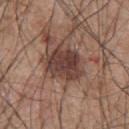Q: Was a biopsy performed?
A: total-body-photography surveillance lesion; no biopsy
Q: What is the lesion's diameter?
A: ≈4.5 mm
Q: What lighting was used for the tile?
A: white-light
Q: Where on the body is the lesion?
A: the upper back
Q: Who is the patient?
A: male, approximately 45 years of age
Q: What kind of image is this?
A: ~15 mm crop, total-body skin-cancer survey